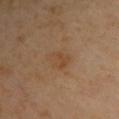Findings:
• follow-up · catalogued during a skin exam; not biopsied
• anatomic site · the upper back
• patient · female, about 50 years old
• image · 15 mm crop, total-body photography